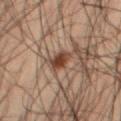Assessment:
The lesion was tiled from a total-body skin photograph and was not biopsied.
Acquisition and patient details:
This is a cross-polarized tile. A male subject aged 48–52. The lesion is on the right thigh. A 15 mm crop from a total-body photograph taken for skin-cancer surveillance.